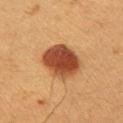follow-up=imaged on a skin check; not biopsied
image=total-body-photography crop, ~15 mm field of view
lesion size=≈5 mm
subject=male, aged 38–42
location=the left upper arm